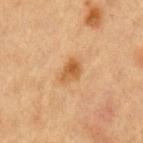Part of a total-body skin-imaging series; this lesion was reviewed on a skin check and was not flagged for biopsy. Automated image analysis of the tile measured a lesion area of about 4.5 mm² and a shape eccentricity near 0.75. The software also gave a normalized lesion–skin contrast near 8. The analysis additionally found a lesion-detection confidence of about 100/100. A female patient, aged approximately 40. Captured under cross-polarized illumination. A 15 mm close-up extracted from a 3D total-body photography capture. The recorded lesion diameter is about 3 mm. Located on the left upper arm.